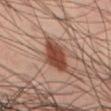Approximately 5 mm at its widest.
On the left thigh.
The subject is a male in their 40s.
A roughly 15 mm field-of-view crop from a total-body skin photograph.